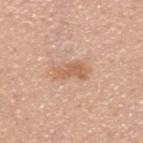An algorithmic analysis of the crop reported a mean CIELAB color near L≈62 a*≈21 b*≈33, roughly 9 lightness units darker than nearby skin, and a normalized border contrast of about 6.5. And it measured a border-irregularity index near 3.5/10, internal color variation of about 2.5 on a 0–10 scale, and radial color variation of about 1. It also reported a classifier nevus-likeness of about 5/100 and a lesion-detection confidence of about 100/100. The lesion is on the upper back. Longest diameter approximately 4 mm. A 15 mm close-up tile from a total-body photography series done for melanoma screening. The patient is a male aged around 40.